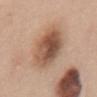biopsy_status: not biopsied; imaged during a skin examination
lesion_size:
  long_diameter_mm_approx: 7.0
image:
  source: total-body photography crop
  field_of_view_mm: 15
lighting: white-light
site: upper back
patient:
  sex: female
  age_approx: 50